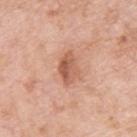A lesion tile, about 15 mm wide, cut from a 3D total-body photograph. Imaged with white-light lighting. Longest diameter approximately 3.5 mm. The lesion-visualizer software estimated a footprint of about 7 mm², an outline eccentricity of about 0.75 (0 = round, 1 = elongated), and two-axis asymmetry of about 0.25. The analysis additionally found a mean CIELAB color near L≈58 a*≈24 b*≈32, a lesion–skin lightness drop of about 11, and a lesion-to-skin contrast of about 7.5 (normalized; higher = more distinct). The analysis additionally found a border-irregularity index near 2.5/10, internal color variation of about 5.5 on a 0–10 scale, and peripheral color asymmetry of about 2. Located on the upper back. A male patient about 60 years old.